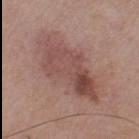  site: right thigh
  lighting: white-light
  image:
    source: total-body photography crop
    field_of_view_mm: 15
  lesion_size:
    long_diameter_mm_approx: 8.5
  patient:
    sex: male
    age_approx: 75